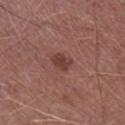Assessment:
The lesion was photographed on a routine skin check and not biopsied; there is no pathology result.
Clinical summary:
Located on the right lower leg. A male subject, in their mid-70s. A 15 mm close-up extracted from a 3D total-body photography capture.